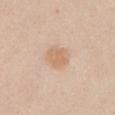| key | value |
|---|---|
| notes | imaged on a skin check; not biopsied |
| image-analysis metrics | border irregularity of about 2.5 on a 0–10 scale, a color-variation rating of about 1.5/10, and a peripheral color-asymmetry measure near 0.5 |
| illumination | white-light |
| image | ~15 mm tile from a whole-body skin photo |
| subject | female, about 40 years old |
| lesion size | about 3 mm |
| anatomic site | the chest |